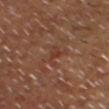Part of a total-body skin-imaging series; this lesion was reviewed on a skin check and was not flagged for biopsy. Cropped from a total-body skin-imaging series; the visible field is about 15 mm. The lesion is located on the head or neck. The lesion-visualizer software estimated an outline eccentricity of about 0.9 (0 = round, 1 = elongated) and a symmetry-axis asymmetry near 0.5. The software also gave an average lesion color of about L≈36 a*≈21 b*≈28 (CIELAB), a lesion–skin lightness drop of about 6, and a normalized lesion–skin contrast near 5.5. Measured at roughly 3 mm in maximum diameter. This is a cross-polarized tile. A male subject, about 60 years old.